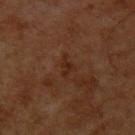- image source: total-body-photography crop, ~15 mm field of view
- site: the upper back
- lesion diameter: ~2.5 mm (longest diameter)
- illumination: cross-polarized
- subject: male, aged around 60